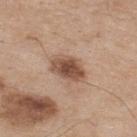biopsy_status: not biopsied; imaged during a skin examination
image:
  source: total-body photography crop
  field_of_view_mm: 15
site: upper back
patient:
  sex: male
  age_approx: 75